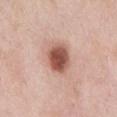This lesion was catalogued during total-body skin photography and was not selected for biopsy.
An algorithmic analysis of the crop reported an average lesion color of about L≈53 a*≈25 b*≈27 (CIELAB), about 17 CIELAB-L* units darker than the surrounding skin, and a normalized lesion–skin contrast near 11. It also reported a border-irregularity rating of about 1/10 and a peripheral color-asymmetry measure near 1.
The lesion is on the abdomen.
Longest diameter approximately 4 mm.
A male subject, about 55 years old.
Cropped from a total-body skin-imaging series; the visible field is about 15 mm.
Captured under white-light illumination.